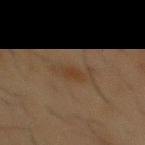  biopsy_status: not biopsied; imaged during a skin examination
  patient:
    sex: male
    age_approx: 55
  image:
    source: total-body photography crop
    field_of_view_mm: 15
  lighting: cross-polarized
  site: front of the torso
  lesion_size:
    long_diameter_mm_approx: 4.5
  automated_metrics:
    area_mm2_approx: 6.0
    eccentricity: 0.95
    shape_asymmetry: 0.35
    cielab_L: 33
    cielab_a: 13
    cielab_b: 26
    vs_skin_contrast_norm: 5.5
    color_variation_0_10: 1.5
    peripheral_color_asymmetry: 0.5